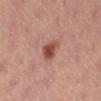Findings:
• workup — imaged on a skin check; not biopsied
• lesion diameter — ≈3 mm
• acquisition — ~15 mm crop, total-body skin-cancer survey
• site — the left lower leg
• tile lighting — white-light illumination
• subject — female, roughly 25 years of age